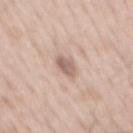The lesion is on the mid back.
A male subject, about 50 years old.
A lesion tile, about 15 mm wide, cut from a 3D total-body photograph.